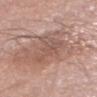  biopsy_status: not biopsied; imaged during a skin examination